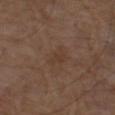Q: What is the imaging modality?
A: total-body-photography crop, ~15 mm field of view
Q: Illumination type?
A: white-light illumination
Q: Patient demographics?
A: male, about 75 years old
Q: Where on the body is the lesion?
A: the left thigh
Q: Lesion size?
A: ~2.5 mm (longest diameter)